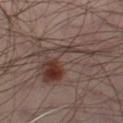A male subject, about 40 years old. A 15 mm crop from a total-body photograph taken for skin-cancer surveillance. From the left thigh.